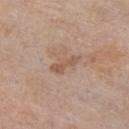biopsy status = imaged on a skin check; not biopsied
image = ~15 mm tile from a whole-body skin photo
subject = female, in their mid-60s
anatomic site = the front of the torso
lighting = white-light illumination
diameter = ≈3.5 mm